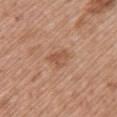Clinical impression: This lesion was catalogued during total-body skin photography and was not selected for biopsy. Acquisition and patient details: Located on the back. The tile uses white-light illumination. A female subject aged 58–62. A lesion tile, about 15 mm wide, cut from a 3D total-body photograph.